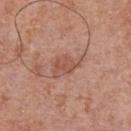biopsy_status: not biopsied; imaged during a skin examination
site: chest
image:
  source: total-body photography crop
  field_of_view_mm: 15
lesion_size:
  long_diameter_mm_approx: 4.0
lighting: white-light
patient:
  sex: male
  age_approx: 70